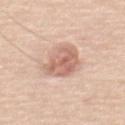Clinical impression:
The lesion was photographed on a routine skin check and not biopsied; there is no pathology result.
Background:
A male subject, aged 58 to 62. A roughly 15 mm field-of-view crop from a total-body skin photograph. Captured under white-light illumination. The lesion-visualizer software estimated an average lesion color of about L≈64 a*≈21 b*≈28 (CIELAB) and about 12 CIELAB-L* units darker than the surrounding skin. The software also gave a nevus-likeness score of about 75/100. Located on the upper back.